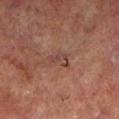{"biopsy_status": "not biopsied; imaged during a skin examination", "lesion_size": {"long_diameter_mm_approx": 3.0}, "lighting": "cross-polarized", "patient": {"sex": "male", "age_approx": 70}, "site": "left lower leg", "automated_metrics": {"area_mm2_approx": 3.5, "eccentricity": 0.85, "border_irregularity_0_10": 5.5, "color_variation_0_10": 1.0}, "image": {"source": "total-body photography crop", "field_of_view_mm": 15}}